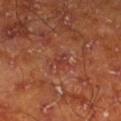notes = no biopsy performed (imaged during a skin exam) | illumination = cross-polarized | image source = total-body-photography crop, ~15 mm field of view | patient = male, aged 63 to 67 | anatomic site = the left lower leg.